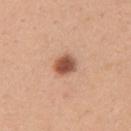Assessment: The lesion was tiled from a total-body skin photograph and was not biopsied. Context: A close-up tile cropped from a whole-body skin photograph, about 15 mm across. The lesion is located on the left upper arm. Automated tile analysis of the lesion measured an area of roughly 5.5 mm², an eccentricity of roughly 0.6, and a symmetry-axis asymmetry near 0.15. And it measured a mean CIELAB color near L≈53 a*≈24 b*≈31, roughly 17 lightness units darker than nearby skin, and a normalized border contrast of about 11. And it measured border irregularity of about 1.5 on a 0–10 scale and a peripheral color-asymmetry measure near 1. The analysis additionally found a lesion-detection confidence of about 100/100. The lesion's longest dimension is about 3 mm. Captured under white-light illumination. The patient is a female aged around 30.